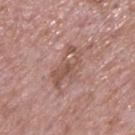{
  "biopsy_status": "not biopsied; imaged during a skin examination",
  "site": "back",
  "lesion_size": {
    "long_diameter_mm_approx": 5.0
  },
  "image": {
    "source": "total-body photography crop",
    "field_of_view_mm": 15
  },
  "patient": {
    "sex": "male",
    "age_approx": 65
  }
}